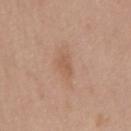follow-up = no biopsy performed (imaged during a skin exam) | patient = male, roughly 50 years of age | imaging modality = 15 mm crop, total-body photography | site = the mid back | automated lesion analysis = an average lesion color of about L≈56 a*≈20 b*≈31 (CIELAB), roughly 7 lightness units darker than nearby skin, and a normalized lesion–skin contrast near 5; a border-irregularity rating of about 3/10, a within-lesion color-variation index near 1/10, and radial color variation of about 0.5; a lesion-detection confidence of about 100/100 | lesion size = ~2.5 mm (longest diameter).